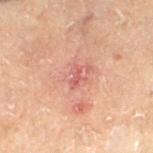No biopsy was performed on this lesion — it was imaged during a full skin examination and was not determined to be concerning. A male subject approximately 70 years of age. A close-up tile cropped from a whole-body skin photograph, about 15 mm across. On the left lower leg.